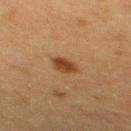  biopsy_status: not biopsied; imaged during a skin examination
  site: upper back
  image:
    source: total-body photography crop
    field_of_view_mm: 15
  patient:
    sex: female
    age_approx: 55